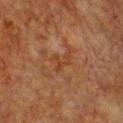No biopsy was performed on this lesion — it was imaged during a full skin examination and was not determined to be concerning. From the chest. A female subject, in their mid- to late 50s. A 15 mm crop from a total-body photograph taken for skin-cancer surveillance. An algorithmic analysis of the crop reported an area of roughly 3.5 mm², an eccentricity of roughly 0.7, and a symmetry-axis asymmetry near 0.7. And it measured a lesion–skin lightness drop of about 5. And it measured internal color variation of about 0 on a 0–10 scale and a peripheral color-asymmetry measure near 0. It also reported a classifier nevus-likeness of about 0/100 and lesion-presence confidence of about 100/100. Longest diameter approximately 3 mm.